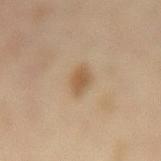Case summary:
- workup — no biopsy performed (imaged during a skin exam)
- TBP lesion metrics — an outline eccentricity of about 0.75 (0 = round, 1 = elongated) and a symmetry-axis asymmetry near 0.35; a classifier nevus-likeness of about 75/100 and a detector confidence of about 100 out of 100 that the crop contains a lesion
- lighting — cross-polarized illumination
- image — 15 mm crop, total-body photography
- body site — the mid back
- subject — female, aged approximately 60